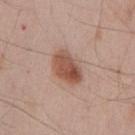anatomic site = the chest | patient = male, roughly 65 years of age | image = 15 mm crop, total-body photography.